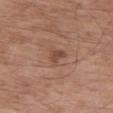Notes:
• patient · male, roughly 55 years of age
• anatomic site · the left thigh
• automated lesion analysis · a lesion area of about 3.5 mm² and a symmetry-axis asymmetry near 0.45; a detector confidence of about 100 out of 100 that the crop contains a lesion
• image · ~15 mm tile from a whole-body skin photo
• illumination · white-light
• lesion diameter · ≈2.5 mm